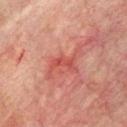workup: catalogued during a skin exam; not biopsied
patient: male, about 70 years old
anatomic site: the chest
imaging modality: ~15 mm crop, total-body skin-cancer survey